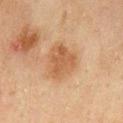Imaged during a routine full-body skin examination; the lesion was not biopsied and no histopathology is available.
On the back.
A male patient aged around 45.
Cropped from a whole-body photographic skin survey; the tile spans about 15 mm.